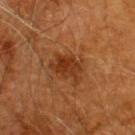Impression: Captured during whole-body skin photography for melanoma surveillance; the lesion was not biopsied. Acquisition and patient details: This is a cross-polarized tile. From the back. Automated image analysis of the tile measured a border-irregularity rating of about 3/10 and internal color variation of about 3.5 on a 0–10 scale. A male subject aged 58–62. A 15 mm close-up extracted from a 3D total-body photography capture.